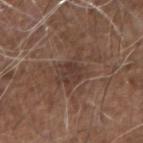Part of a total-body skin-imaging series; this lesion was reviewed on a skin check and was not flagged for biopsy. A male patient, about 75 years old. The tile uses white-light illumination. The lesion is located on the left forearm. A 15 mm crop from a total-body photograph taken for skin-cancer surveillance. The lesion-visualizer software estimated a peripheral color-asymmetry measure near 1. The analysis additionally found a nevus-likeness score of about 0/100 and lesion-presence confidence of about 90/100.